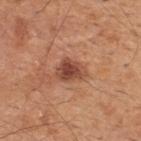Recorded during total-body skin imaging; not selected for excision or biopsy. Longest diameter approximately 3.5 mm. Located on the back. Automated image analysis of the tile measured an area of roughly 7 mm², an outline eccentricity of about 0.65 (0 = round, 1 = elongated), and a symmetry-axis asymmetry near 0.3. A male subject, in their mid-40s. This is a white-light tile. Cropped from a total-body skin-imaging series; the visible field is about 15 mm.